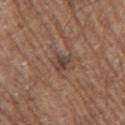Case summary:
* follow-up · catalogued during a skin exam; not biopsied
* lighting · white-light
* lesion size · ~3 mm (longest diameter)
* subject · male, aged approximately 70
* location · the right upper arm
* imaging modality · ~15 mm crop, total-body skin-cancer survey